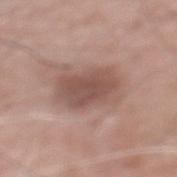The lesion was tiled from a total-body skin photograph and was not biopsied. The lesion is located on the mid back. Captured under white-light illumination. A close-up tile cropped from a whole-body skin photograph, about 15 mm across. The lesion-visualizer software estimated a lesion color around L≈52 a*≈19 b*≈23 in CIELAB and a lesion–skin lightness drop of about 11. The analysis additionally found lesion-presence confidence of about 100/100. The recorded lesion diameter is about 5.5 mm. A male subject, in their 60s.